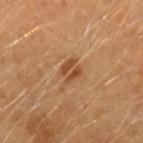Part of a total-body skin-imaging series; this lesion was reviewed on a skin check and was not flagged for biopsy. Imaged with cross-polarized lighting. Approximately 2.5 mm at its widest. Automated image analysis of the tile measured a lesion area of about 5.5 mm², an outline eccentricity of about 0.45 (0 = round, 1 = elongated), and a shape-asymmetry score of about 0.2 (0 = symmetric). It also reported a lesion color around L≈50 a*≈23 b*≈37 in CIELAB, about 10 CIELAB-L* units darker than the surrounding skin, and a lesion-to-skin contrast of about 7 (normalized; higher = more distinct). And it measured a border-irregularity rating of about 2/10 and a within-lesion color-variation index near 4.5/10. And it measured an automated nevus-likeness rating near 25 out of 100 and a lesion-detection confidence of about 100/100. Located on the left forearm. Cropped from a total-body skin-imaging series; the visible field is about 15 mm. A male patient aged approximately 40.